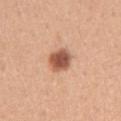Impression: Recorded during total-body skin imaging; not selected for excision or biopsy. Background: An algorithmic analysis of the crop reported an eccentricity of roughly 0.3 and two-axis asymmetry of about 0.15. And it measured a mean CIELAB color near L≈56 a*≈24 b*≈32 and a lesion–skin lightness drop of about 16. The lesion is located on the left upper arm. The patient is a female roughly 30 years of age. Cropped from a whole-body photographic skin survey; the tile spans about 15 mm. Imaged with white-light lighting. Measured at roughly 3 mm in maximum diameter.